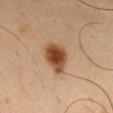{
  "biopsy_status": "not biopsied; imaged during a skin examination",
  "patient": {
    "sex": "male",
    "age_approx": 50
  },
  "site": "left thigh",
  "image": {
    "source": "total-body photography crop",
    "field_of_view_mm": 15
  },
  "lesion_size": {
    "long_diameter_mm_approx": 4.0
  }
}